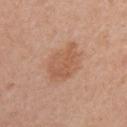Q: Was this lesion biopsied?
A: catalogued during a skin exam; not biopsied
Q: Patient demographics?
A: female, in their mid- to late 50s
Q: Illumination type?
A: white-light
Q: What is the anatomic site?
A: the right upper arm
Q: What is the lesion's diameter?
A: ≈5 mm
Q: What kind of image is this?
A: total-body-photography crop, ~15 mm field of view